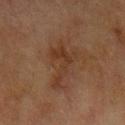Recorded during total-body skin imaging; not selected for excision or biopsy.